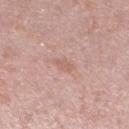The lesion was tiled from a total-body skin photograph and was not biopsied. The lesion is on the left lower leg. A female patient, about 40 years old. A 15 mm crop from a total-body photograph taken for skin-cancer surveillance.